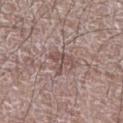Clinical impression: Part of a total-body skin-imaging series; this lesion was reviewed on a skin check and was not flagged for biopsy. Context: Imaged with white-light lighting. The lesion-visualizer software estimated a lesion color around L≈50 a*≈17 b*≈20 in CIELAB, roughly 9 lightness units darker than nearby skin, and a normalized border contrast of about 6.5. The analysis additionally found border irregularity of about 5.5 on a 0–10 scale, a within-lesion color-variation index near 3.5/10, and radial color variation of about 1.5. It also reported an automated nevus-likeness rating near 0 out of 100. A lesion tile, about 15 mm wide, cut from a 3D total-body photograph. The subject is a male aged around 65. Located on the leg. Measured at roughly 3.5 mm in maximum diameter.